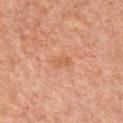From the chest.
The tile uses cross-polarized illumination.
The subject is a male about 60 years old.
A 15 mm close-up tile from a total-body photography series done for melanoma screening.
The recorded lesion diameter is about 3 mm.